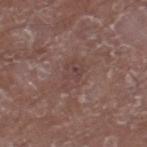Q: Is there a histopathology result?
A: imaged on a skin check; not biopsied
Q: Patient demographics?
A: male, aged 63–67
Q: Automated lesion metrics?
A: an area of roughly 6.5 mm², an outline eccentricity of about 0.8 (0 = round, 1 = elongated), and a symmetry-axis asymmetry near 0.25; a mean CIELAB color near L≈42 a*≈18 b*≈20, a lesion–skin lightness drop of about 6, and a lesion-to-skin contrast of about 5 (normalized; higher = more distinct); a border-irregularity rating of about 3/10, a within-lesion color-variation index near 3.5/10, and a peripheral color-asymmetry measure near 1
Q: Where on the body is the lesion?
A: the left thigh
Q: What is the lesion's diameter?
A: about 3.5 mm
Q: Illumination type?
A: white-light
Q: What is the imaging modality?
A: total-body-photography crop, ~15 mm field of view